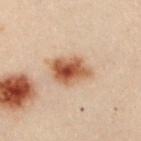Assessment: This lesion was catalogued during total-body skin photography and was not selected for biopsy. Context: A female subject aged 28 to 32. Cropped from a total-body skin-imaging series; the visible field is about 15 mm. From the chest.